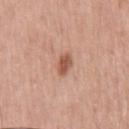workup — no biopsy performed (imaged during a skin exam) | subject — male, in their 60s | acquisition — ~15 mm tile from a whole-body skin photo | site — the front of the torso.